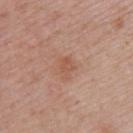The lesion was photographed on a routine skin check and not biopsied; there is no pathology result.
A female subject roughly 50 years of age.
Located on the upper back.
A lesion tile, about 15 mm wide, cut from a 3D total-body photograph.